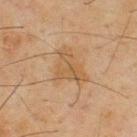Assessment: The lesion was tiled from a total-body skin photograph and was not biopsied. Context: The lesion-visualizer software estimated a shape eccentricity near 0.65 and a symmetry-axis asymmetry near 0.55. It also reported border irregularity of about 6 on a 0–10 scale, internal color variation of about 3 on a 0–10 scale, and radial color variation of about 1. The analysis additionally found a nevus-likeness score of about 0/100 and a lesion-detection confidence of about 100/100. Cropped from a total-body skin-imaging series; the visible field is about 15 mm. Located on the upper back. A male patient, aged 43 to 47.